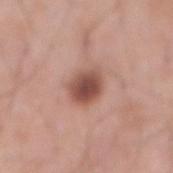workup: catalogued during a skin exam; not biopsied
automated lesion analysis: a mean CIELAB color near L≈50 a*≈23 b*≈26, about 14 CIELAB-L* units darker than the surrounding skin, and a normalized lesion–skin contrast near 10; border irregularity of about 1.5 on a 0–10 scale and radial color variation of about 1
imaging modality: ~15 mm crop, total-body skin-cancer survey
illumination: white-light
subject: male, about 70 years old
location: the front of the torso